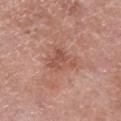workup: total-body-photography surveillance lesion; no biopsy
acquisition: ~15 mm crop, total-body skin-cancer survey
patient: male, aged 68 to 72
site: the leg